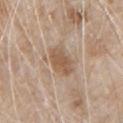Recorded during total-body skin imaging; not selected for excision or biopsy. A roughly 15 mm field-of-view crop from a total-body skin photograph. The recorded lesion diameter is about 4 mm. A male subject, roughly 80 years of age. On the chest.